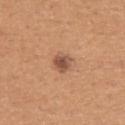Captured during whole-body skin photography for melanoma surveillance; the lesion was not biopsied. Located on the left upper arm. A region of skin cropped from a whole-body photographic capture, roughly 15 mm wide. A female patient, approximately 40 years of age.